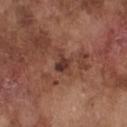Case summary:
* image source — 15 mm crop, total-body photography
* tile lighting — white-light
* lesion size — ≈3 mm
* anatomic site — the chest
* patient — male, aged 73 to 77